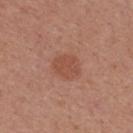  biopsy_status: not biopsied; imaged during a skin examination